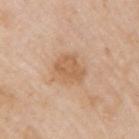biopsy status: no biopsy performed (imaged during a skin exam) | body site: the right upper arm | illumination: white-light illumination | size: ≈3.5 mm | subject: male, in their mid- to late 70s | image source: total-body-photography crop, ~15 mm field of view.